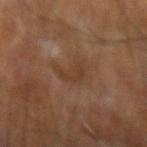<lesion>
<biopsy_status>not biopsied; imaged during a skin examination</biopsy_status>
<lesion_size>
  <long_diameter_mm_approx>3.5</long_diameter_mm_approx>
</lesion_size>
<site>right forearm</site>
<lighting>cross-polarized</lighting>
<patient>
  <sex>male</sex>
  <age_approx>65</age_approx>
</patient>
<image>
  <source>total-body photography crop</source>
  <field_of_view_mm>15</field_of_view_mm>
</image>
</lesion>